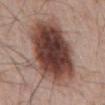Recorded during total-body skin imaging; not selected for excision or biopsy. A male patient, aged around 65. On the abdomen. A 15 mm close-up tile from a total-body photography series done for melanoma screening.